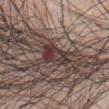No biopsy was performed on this lesion — it was imaged during a full skin examination and was not determined to be concerning.
A 15 mm close-up extracted from a 3D total-body photography capture.
Measured at roughly 4 mm in maximum diameter.
Located on the front of the torso.
A male patient, in their 70s.